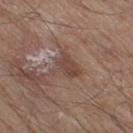Clinical impression:
Imaged during a routine full-body skin examination; the lesion was not biopsied and no histopathology is available.
Image and clinical context:
A lesion tile, about 15 mm wide, cut from a 3D total-body photograph. Approximately 3.5 mm at its widest. This is a white-light tile. The patient is a male about 80 years old. Located on the right thigh.